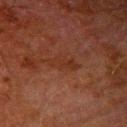{
  "biopsy_status": "not biopsied; imaged during a skin examination",
  "image": {
    "source": "total-body photography crop",
    "field_of_view_mm": 15
  },
  "site": "right upper arm",
  "patient": {
    "sex": "male",
    "age_approx": 80
  }
}